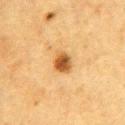Impression:
Captured during whole-body skin photography for melanoma surveillance; the lesion was not biopsied.
Context:
On the chest. Longest diameter approximately 2.5 mm. A region of skin cropped from a whole-body photographic capture, roughly 15 mm wide. A female patient, aged 53 to 57. This is a cross-polarized tile.